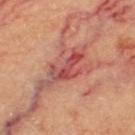• follow-up · imaged on a skin check; not biopsied
• imaging modality · ~15 mm tile from a whole-body skin photo
• lighting · cross-polarized illumination
• diameter · ≈5 mm
• subject · female, aged 58–62
• TBP lesion metrics · an area of roughly 8.5 mm²; about 11 CIELAB-L* units darker than the surrounding skin and a lesion-to-skin contrast of about 8 (normalized; higher = more distinct); a border-irregularity index near 7/10, internal color variation of about 8 on a 0–10 scale, and a peripheral color-asymmetry measure near 2.5; a classifier nevus-likeness of about 0/100 and lesion-presence confidence of about 100/100
• anatomic site · the left thigh